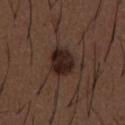<tbp_lesion>
<biopsy_status>not biopsied; imaged during a skin examination</biopsy_status>
<patient>
  <sex>male</sex>
  <age_approx>50</age_approx>
</patient>
<lighting>white-light</lighting>
<site>abdomen</site>
<image>
  <source>total-body photography crop</source>
  <field_of_view_mm>15</field_of_view_mm>
</image>
<lesion_size>
  <long_diameter_mm_approx>4.0</long_diameter_mm_approx>
</lesion_size>
<automated_metrics>
  <vs_skin_contrast_norm>13.0</vs_skin_contrast_norm>
  <lesion_detection_confidence_0_100>100</lesion_detection_confidence_0_100>
</automated_metrics>
</tbp_lesion>